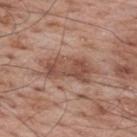Q: Lesion size?
A: about 6.5 mm
Q: How was this image acquired?
A: 15 mm crop, total-body photography
Q: Where on the body is the lesion?
A: the upper back
Q: Illumination type?
A: white-light illumination
Q: Automated lesion metrics?
A: an average lesion color of about L≈50 a*≈21 b*≈27 (CIELAB), roughly 10 lightness units darker than nearby skin, and a normalized border contrast of about 7; border irregularity of about 3.5 on a 0–10 scale, internal color variation of about 4 on a 0–10 scale, and peripheral color asymmetry of about 1.5; an automated nevus-likeness rating near 0 out of 100 and a detector confidence of about 100 out of 100 that the crop contains a lesion
Q: Who is the patient?
A: male, aged 68–72